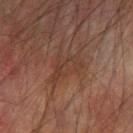image source — ~15 mm crop, total-body skin-cancer survey
location — the left forearm
illumination — cross-polarized
patient — male, roughly 75 years of age
lesion size — ~4 mm (longest diameter)